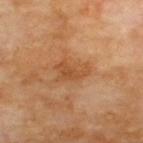Q: Was a biopsy performed?
A: catalogued during a skin exam; not biopsied
Q: Where on the body is the lesion?
A: the upper back
Q: Illumination type?
A: cross-polarized illumination
Q: What kind of image is this?
A: total-body-photography crop, ~15 mm field of view
Q: Lesion size?
A: ~4 mm (longest diameter)
Q: Automated lesion metrics?
A: an area of roughly 7 mm² and an eccentricity of roughly 0.8; border irregularity of about 4.5 on a 0–10 scale and a peripheral color-asymmetry measure near 1
Q: Who is the patient?
A: male, about 70 years old